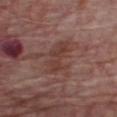notes=imaged on a skin check; not biopsied
location=the chest
tile lighting=white-light illumination
patient=male, aged around 65
imaging modality=~15 mm tile from a whole-body skin photo